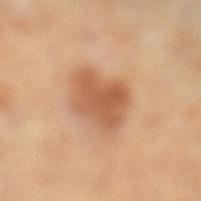biopsy_status: not biopsied; imaged during a skin examination
image:
  source: total-body photography crop
  field_of_view_mm: 15
automated_metrics:
  area_mm2_approx: 16.0
  eccentricity: 0.65
  shape_asymmetry: 0.2
  border_irregularity_0_10: 2.5
  color_variation_0_10: 3.5
  peripheral_color_asymmetry: 1.0
  nevus_likeness_0_100: 85
  lesion_detection_confidence_0_100: 100
site: right leg
lesion_size:
  long_diameter_mm_approx: 5.0
patient:
  sex: female
  age_approx: 65
lighting: cross-polarized